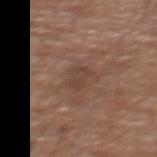The lesion was photographed on a routine skin check and not biopsied; there is no pathology result. A region of skin cropped from a whole-body photographic capture, roughly 15 mm wide. From the upper back. About 2.5 mm across. Imaged with white-light lighting. A male subject, aged approximately 75.